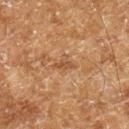The lesion was photographed on a routine skin check and not biopsied; there is no pathology result. Automated tile analysis of the lesion measured an area of roughly 2.5 mm², an outline eccentricity of about 0.9 (0 = round, 1 = elongated), and a shape-asymmetry score of about 0.35 (0 = symmetric). And it measured a mean CIELAB color near L≈38 a*≈17 b*≈29 and roughly 7 lightness units darker than nearby skin. The software also gave border irregularity of about 4 on a 0–10 scale, a within-lesion color-variation index near 0.5/10, and peripheral color asymmetry of about 0. A 15 mm close-up extracted from a 3D total-body photography capture. The patient is a male aged 58–62. The lesion's longest dimension is about 3 mm. Captured under cross-polarized illumination. From the right lower leg.